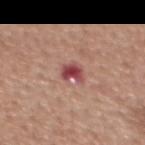Captured during whole-body skin photography for melanoma surveillance; the lesion was not biopsied.
A female subject aged 48–52.
On the upper back.
A region of skin cropped from a whole-body photographic capture, roughly 15 mm wide.
About 3 mm across.